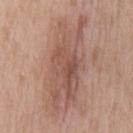follow-up: imaged on a skin check; not biopsied | illumination: white-light | body site: the chest | subject: male, roughly 50 years of age | image: 15 mm crop, total-body photography | TBP lesion metrics: a mean CIELAB color near L≈53 a*≈20 b*≈26, roughly 10 lightness units darker than nearby skin, and a lesion-to-skin contrast of about 7 (normalized; higher = more distinct); an automated nevus-likeness rating near 35 out of 100 and a detector confidence of about 95 out of 100 that the crop contains a lesion | diameter: ~11.5 mm (longest diameter).